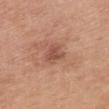Part of a total-body skin-imaging series; this lesion was reviewed on a skin check and was not flagged for biopsy.
A female patient, in their mid- to late 50s.
This is a white-light tile.
Automated tile analysis of the lesion measured a footprint of about 4.5 mm² and a symmetry-axis asymmetry near 0.4. It also reported a lesion color around L≈51 a*≈24 b*≈29 in CIELAB, about 9 CIELAB-L* units darker than the surrounding skin, and a normalized border contrast of about 6.5. And it measured a border-irregularity rating of about 4.5/10, a color-variation rating of about 2.5/10, and a peripheral color-asymmetry measure near 1. It also reported a nevus-likeness score of about 0/100.
From the chest.
Cropped from a whole-body photographic skin survey; the tile spans about 15 mm.